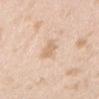This lesion was catalogued during total-body skin photography and was not selected for biopsy.
Longest diameter approximately 3 mm.
An algorithmic analysis of the crop reported a border-irregularity index near 3/10 and peripheral color asymmetry of about 0.
On the head or neck.
This image is a 15 mm lesion crop taken from a total-body photograph.
A female patient, in their mid-20s.
This is a white-light tile.